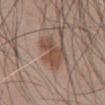Automated image analysis of the tile measured a lesion area of about 9.5 mm² and an outline eccentricity of about 0.65 (0 = round, 1 = elongated). The analysis additionally found about 9 CIELAB-L* units darker than the surrounding skin and a lesion-to-skin contrast of about 7 (normalized; higher = more distinct). The analysis additionally found internal color variation of about 2.5 on a 0–10 scale. The lesion's longest dimension is about 4.5 mm. From the abdomen. A male patient, about 50 years old. A region of skin cropped from a whole-body photographic capture, roughly 15 mm wide. This is a white-light tile.